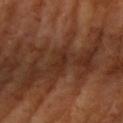Impression: The lesion was photographed on a routine skin check and not biopsied; there is no pathology result. Acquisition and patient details: An algorithmic analysis of the crop reported a lesion area of about 2 mm², an eccentricity of roughly 0.9, and two-axis asymmetry of about 0.35. And it measured a border-irregularity index near 4/10. And it measured lesion-presence confidence of about 75/100. The lesion is on the right upper arm. The patient is a male aged around 65. Imaged with cross-polarized lighting. The lesion's longest dimension is about 2.5 mm. A region of skin cropped from a whole-body photographic capture, roughly 15 mm wide.